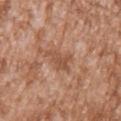{"patient": {"sex": "male", "age_approx": 45}, "lighting": "white-light", "automated_metrics": {"border_irregularity_0_10": 5.5, "color_variation_0_10": 2.0, "peripheral_color_asymmetry": 0.5, "nevus_likeness_0_100": 0, "lesion_detection_confidence_0_100": 100}, "site": "left upper arm", "image": {"source": "total-body photography crop", "field_of_view_mm": 15}}